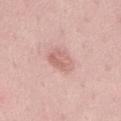Imaged during a routine full-body skin examination; the lesion was not biopsied and no histopathology is available.
The lesion-visualizer software estimated a lesion area of about 6.5 mm² and a symmetry-axis asymmetry near 0.2. And it measured a border-irregularity rating of about 2/10, a color-variation rating of about 3.5/10, and a peripheral color-asymmetry measure near 1.5.
Cropped from a whole-body photographic skin survey; the tile spans about 15 mm.
The lesion is located on the chest.
This is a white-light tile.
A male subject aged 48–52.
The lesion's longest dimension is about 3.5 mm.